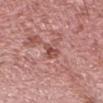Acquisition and patient details:
A region of skin cropped from a whole-body photographic capture, roughly 15 mm wide. Imaged with white-light lighting. The recorded lesion diameter is about 3.5 mm. The lesion is on the head or neck. Automated tile analysis of the lesion measured an average lesion color of about L≈50 a*≈27 b*≈25 (CIELAB) and a lesion–skin lightness drop of about 10. The software also gave a nevus-likeness score of about 0/100. The subject is a male approximately 65 years of age.